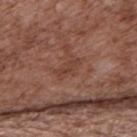Imaged during a routine full-body skin examination; the lesion was not biopsied and no histopathology is available. The lesion is located on the upper back. Captured under white-light illumination. Automated image analysis of the tile measured an area of roughly 4 mm², a shape eccentricity near 0.9, and two-axis asymmetry of about 0.3. And it measured a lesion color around L≈41 a*≈22 b*≈27 in CIELAB, roughly 6 lightness units darker than nearby skin, and a normalized lesion–skin contrast near 5.5. And it measured a classifier nevus-likeness of about 0/100 and a lesion-detection confidence of about 100/100. Longest diameter approximately 3.5 mm. A male subject in their mid-70s. A close-up tile cropped from a whole-body skin photograph, about 15 mm across.